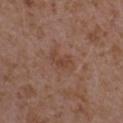biopsy status = total-body-photography surveillance lesion; no biopsy
location = the left upper arm
acquisition = ~15 mm tile from a whole-body skin photo
tile lighting = white-light
automated lesion analysis = a lesion area of about 4.5 mm², a shape eccentricity near 0.85, and two-axis asymmetry of about 0.3; border irregularity of about 3.5 on a 0–10 scale and a within-lesion color-variation index near 1.5/10; lesion-presence confidence of about 100/100
lesion size = ~3.5 mm (longest diameter)
subject = female, in their mid-30s